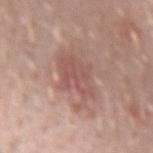anatomic site — the left forearm
patient — male, about 30 years old
lesion diameter — ~6.5 mm (longest diameter)
imaging modality — ~15 mm crop, total-body skin-cancer survey
automated metrics — a footprint of about 15 mm² and an eccentricity of roughly 0.85; a border-irregularity index near 6/10, a color-variation rating of about 3/10, and a peripheral color-asymmetry measure near 1; a nevus-likeness score of about 0/100 and a lesion-detection confidence of about 100/100
tile lighting — white-light